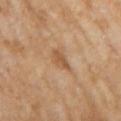workup — total-body-photography surveillance lesion; no biopsy | image source — ~15 mm crop, total-body skin-cancer survey | lighting — cross-polarized illumination | patient — female, roughly 60 years of age | body site — the right upper arm | lesion size — ≈3 mm.